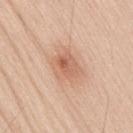Q: Is there a histopathology result?
A: catalogued during a skin exam; not biopsied
Q: Patient demographics?
A: male, in their 60s
Q: How was this image acquired?
A: ~15 mm tile from a whole-body skin photo
Q: What did automated image analysis measure?
A: an average lesion color of about L≈63 a*≈22 b*≈32 (CIELAB), roughly 9 lightness units darker than nearby skin, and a lesion-to-skin contrast of about 6 (normalized; higher = more distinct); border irregularity of about 2.5 on a 0–10 scale, a color-variation rating of about 6/10, and peripheral color asymmetry of about 2
Q: Where on the body is the lesion?
A: the lower back